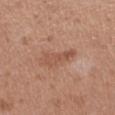* notes: imaged on a skin check; not biopsied
* imaging modality: ~15 mm crop, total-body skin-cancer survey
* illumination: white-light
* subject: female, aged 38 to 42
* body site: the left thigh